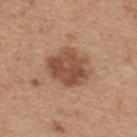| key | value |
|---|---|
| biopsy status | no biopsy performed (imaged during a skin exam) |
| lesion diameter | about 5 mm |
| subject | male, about 65 years old |
| body site | the back |
| acquisition | total-body-photography crop, ~15 mm field of view |